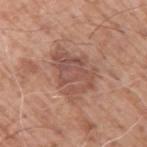Captured during whole-body skin photography for melanoma surveillance; the lesion was not biopsied. A 15 mm crop from a total-body photograph taken for skin-cancer surveillance. This is a white-light tile. From the arm. Approximately 5.5 mm at its widest. A male patient, in their 60s.